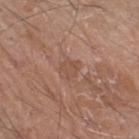Recorded during total-body skin imaging; not selected for excision or biopsy.
A 15 mm close-up tile from a total-body photography series done for melanoma screening.
Captured under white-light illumination.
Longest diameter approximately 2.5 mm.
A male patient, aged 58–62.
The lesion-visualizer software estimated an average lesion color of about L≈50 a*≈20 b*≈28 (CIELAB) and a lesion-to-skin contrast of about 4.5 (normalized; higher = more distinct). The software also gave border irregularity of about 2 on a 0–10 scale, a within-lesion color-variation index near 1/10, and a peripheral color-asymmetry measure near 0.5. And it measured a nevus-likeness score of about 0/100 and a detector confidence of about 100 out of 100 that the crop contains a lesion.
The lesion is located on the leg.